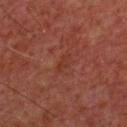No biopsy was performed on this lesion — it was imaged during a full skin examination and was not determined to be concerning.
On the chest.
The tile uses cross-polarized illumination.
A 15 mm close-up tile from a total-body photography series done for melanoma screening.
A male subject aged around 60.
An algorithmic analysis of the crop reported a footprint of about 2.5 mm², a shape eccentricity near 0.85, and a shape-asymmetry score of about 0.35 (0 = symmetric). The analysis additionally found a lesion color around L≈32 a*≈25 b*≈27 in CIELAB, about 4 CIELAB-L* units darker than the surrounding skin, and a normalized border contrast of about 5. The analysis additionally found a border-irregularity rating of about 3.5/10, a color-variation rating of about 0/10, and a peripheral color-asymmetry measure near 0.